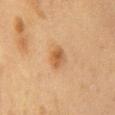workup: total-body-photography surveillance lesion; no biopsy
diameter: ~2.5 mm (longest diameter)
illumination: cross-polarized
subject: female, approximately 50 years of age
acquisition: ~15 mm tile from a whole-body skin photo
body site: the front of the torso
automated lesion analysis: an average lesion color of about L≈46 a*≈18 b*≈33 (CIELAB) and a normalized lesion–skin contrast near 7.5; a border-irregularity index near 1.5/10 and a peripheral color-asymmetry measure near 1